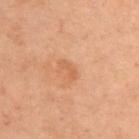{"biopsy_status": "not biopsied; imaged during a skin examination"}